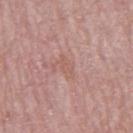follow-up: no biopsy performed (imaged during a skin exam) | image: total-body-photography crop, ~15 mm field of view | body site: the right thigh | lesion diameter: ~3 mm (longest diameter) | patient: female, aged around 65 | lighting: white-light.